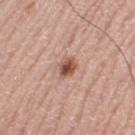Captured during whole-body skin photography for melanoma surveillance; the lesion was not biopsied. The lesion is on the left thigh. Cropped from a whole-body photographic skin survey; the tile spans about 15 mm. The lesion's longest dimension is about 2.5 mm. A male subject aged 68 to 72.